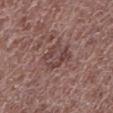Q: Was this lesion biopsied?
A: imaged on a skin check; not biopsied
Q: What did automated image analysis measure?
A: a border-irregularity rating of about 3.5/10, a within-lesion color-variation index near 4.5/10, and radial color variation of about 1.5; a nevus-likeness score of about 0/100
Q: Illumination type?
A: white-light
Q: Patient demographics?
A: male, in their 70s
Q: How was this image acquired?
A: total-body-photography crop, ~15 mm field of view
Q: What is the anatomic site?
A: the left lower leg